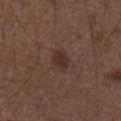| feature | finding |
|---|---|
| subject | male, aged 48–52 |
| acquisition | 15 mm crop, total-body photography |
| site | the right forearm |
| tile lighting | white-light |
| size | ~2.5 mm (longest diameter) |